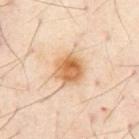The lesion was photographed on a routine skin check and not biopsied; there is no pathology result. The subject is a male aged 53 to 57. The lesion-visualizer software estimated a footprint of about 9.5 mm², a shape eccentricity near 0.3, and a shape-asymmetry score of about 0.1 (0 = symmetric). The analysis additionally found a border-irregularity index near 1.5/10, a color-variation rating of about 6/10, and peripheral color asymmetry of about 2. The analysis additionally found a classifier nevus-likeness of about 100/100 and lesion-presence confidence of about 100/100. The lesion is located on the chest. A 15 mm close-up extracted from a 3D total-body photography capture.